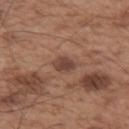  biopsy_status: not biopsied; imaged during a skin examination
  site: left upper arm
  image:
    source: total-body photography crop
    field_of_view_mm: 15
  lesion_size:
    long_diameter_mm_approx: 2.5
  patient:
    sex: male
    age_approx: 55
  lighting: white-light